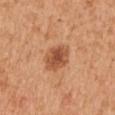Assessment:
The lesion was tiled from a total-body skin photograph and was not biopsied.
Image and clinical context:
Cropped from a whole-body photographic skin survey; the tile spans about 15 mm. The total-body-photography lesion software estimated an area of roughly 8.5 mm². And it measured an average lesion color of about L≈53 a*≈25 b*≈37 (CIELAB) and a normalized lesion–skin contrast near 8.5. The software also gave lesion-presence confidence of about 100/100. This is a white-light tile. Longest diameter approximately 3.5 mm. From the right upper arm. The subject is a male about 55 years old.